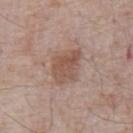{"biopsy_status": "not biopsied; imaged during a skin examination", "patient": {"sex": "male", "age_approx": 70}, "site": "abdomen", "lighting": "white-light", "image": {"source": "total-body photography crop", "field_of_view_mm": 15}, "automated_metrics": {"border_irregularity_0_10": 2.5, "color_variation_0_10": 3.0, "lesion_detection_confidence_0_100": 100}, "lesion_size": {"long_diameter_mm_approx": 4.5}}